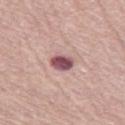<lesion>
<biopsy_status>not biopsied; imaged during a skin examination</biopsy_status>
<patient>
  <sex>female</sex>
  <age_approx>65</age_approx>
</patient>
<image>
  <source>total-body photography crop</source>
  <field_of_view_mm>15</field_of_view_mm>
</image>
<site>left thigh</site>
<lighting>white-light</lighting>
<automated_metrics>
  <nevus_likeness_0_100>10</nevus_likeness_0_100>
  <lesion_detection_confidence_0_100>100</lesion_detection_confidence_0_100>
</automated_metrics>
</lesion>